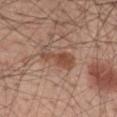Captured during whole-body skin photography for melanoma surveillance; the lesion was not biopsied. Located on the mid back. A 15 mm close-up tile from a total-body photography series done for melanoma screening. Captured under white-light illumination. The patient is a male roughly 60 years of age.